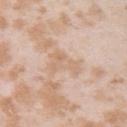{
  "biopsy_status": "not biopsied; imaged during a skin examination"
}